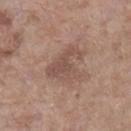Part of a total-body skin-imaging series; this lesion was reviewed on a skin check and was not flagged for biopsy. Approximately 5 mm at its widest. On the left lower leg. A female patient, aged 83 or over. A 15 mm crop from a total-body photograph taken for skin-cancer surveillance. Imaged with white-light lighting.